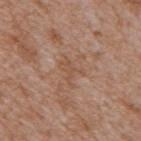Q: Was a biopsy performed?
A: no biopsy performed (imaged during a skin exam)
Q: How large is the lesion?
A: ~3.5 mm (longest diameter)
Q: How was the tile lit?
A: white-light
Q: Automated lesion metrics?
A: a lesion color around L≈52 a*≈19 b*≈30 in CIELAB, roughly 6 lightness units darker than nearby skin, and a normalized border contrast of about 5; a border-irregularity rating of about 8.5/10 and radial color variation of about 0.5; a nevus-likeness score of about 0/100 and a lesion-detection confidence of about 95/100
Q: Where on the body is the lesion?
A: the back
Q: What kind of image is this?
A: ~15 mm crop, total-body skin-cancer survey
Q: What are the patient's age and sex?
A: male, aged 63–67